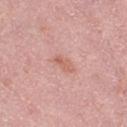Impression: Recorded during total-body skin imaging; not selected for excision or biopsy. Image and clinical context: A female subject aged 38 to 42. The tile uses white-light illumination. The lesion is on the right thigh. A region of skin cropped from a whole-body photographic capture, roughly 15 mm wide. An algorithmic analysis of the crop reported an average lesion color of about L≈62 a*≈25 b*≈27 (CIELAB), roughly 8 lightness units darker than nearby skin, and a normalized border contrast of about 5.5. And it measured a classifier nevus-likeness of about 0/100 and a detector confidence of about 100 out of 100 that the crop contains a lesion.